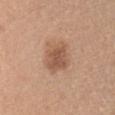Notes:
– workup · imaged on a skin check; not biopsied
– image · ~15 mm tile from a whole-body skin photo
– illumination · white-light illumination
– automated lesion analysis · an eccentricity of roughly 0.5 and a shape-asymmetry score of about 0.25 (0 = symmetric); peripheral color asymmetry of about 1
– anatomic site · the chest
– lesion size · ~4 mm (longest diameter)
– subject · female, aged approximately 30